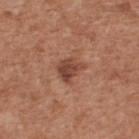The tile uses white-light illumination. A male subject, roughly 65 years of age. Located on the back. A region of skin cropped from a whole-body photographic capture, roughly 15 mm wide.